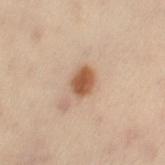follow-up = total-body-photography surveillance lesion; no biopsy
diameter = ~3.5 mm (longest diameter)
patient = female, in their 50s
tile lighting = cross-polarized illumination
acquisition = 15 mm crop, total-body photography
image-analysis metrics = a lesion-detection confidence of about 100/100
location = the leg